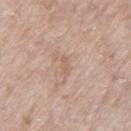Case summary:
- workup — catalogued during a skin exam; not biopsied